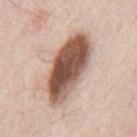The lesion was tiled from a total-body skin photograph and was not biopsied. The subject is a male approximately 80 years of age. The lesion is located on the mid back. A lesion tile, about 15 mm wide, cut from a 3D total-body photograph.